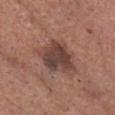– biopsy status — total-body-photography surveillance lesion; no biopsy
– size — about 5 mm
– acquisition — total-body-photography crop, ~15 mm field of view
– location — the head or neck
– patient — male, in their mid-70s